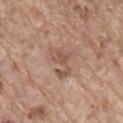This lesion was catalogued during total-body skin photography and was not selected for biopsy. Cropped from a whole-body photographic skin survey; the tile spans about 15 mm. Located on the lower back. Longest diameter approximately 4.5 mm. Imaged with white-light lighting. A male subject, approximately 80 years of age.